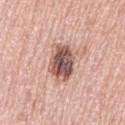Imaged during a routine full-body skin examination; the lesion was not biopsied and no histopathology is available.
The recorded lesion diameter is about 5 mm.
A female patient, aged around 60.
Captured under white-light illumination.
Cropped from a total-body skin-imaging series; the visible field is about 15 mm.
Located on the left thigh.